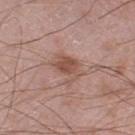The lesion was tiled from a total-body skin photograph and was not biopsied.
From the left thigh.
A male subject roughly 60 years of age.
A close-up tile cropped from a whole-body skin photograph, about 15 mm across.
This is a white-light tile.